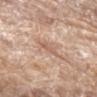Assessment:
No biopsy was performed on this lesion — it was imaged during a full skin examination and was not determined to be concerning.
Image and clinical context:
Captured under white-light illumination. A 15 mm close-up tile from a total-body photography series done for melanoma screening. Located on the left upper arm. A male patient, roughly 80 years of age. An algorithmic analysis of the crop reported border irregularity of about 3.5 on a 0–10 scale, internal color variation of about 1 on a 0–10 scale, and radial color variation of about 0.5. The analysis additionally found an automated nevus-likeness rating near 0 out of 100 and a detector confidence of about 100 out of 100 that the crop contains a lesion.